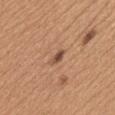Clinical summary: An algorithmic analysis of the crop reported border irregularity of about 3 on a 0–10 scale and a peripheral color-asymmetry measure near 0. And it measured a classifier nevus-likeness of about 20/100 and lesion-presence confidence of about 100/100. Located on the mid back. A female subject, aged around 40. Measured at roughly 3 mm in maximum diameter. The tile uses white-light illumination. A close-up tile cropped from a whole-body skin photograph, about 15 mm across.